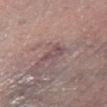Q: Is there a histopathology result?
A: imaged on a skin check; not biopsied
Q: What is the lesion's diameter?
A: about 2.5 mm
Q: Who is the patient?
A: male, aged around 70
Q: Lesion location?
A: the left lower leg
Q: How was the tile lit?
A: white-light illumination
Q: What did automated image analysis measure?
A: a symmetry-axis asymmetry near 0.4; a border-irregularity index near 4/10, internal color variation of about 1.5 on a 0–10 scale, and a peripheral color-asymmetry measure near 0.5; a classifier nevus-likeness of about 0/100 and a detector confidence of about 55 out of 100 that the crop contains a lesion
Q: How was this image acquired?
A: 15 mm crop, total-body photography